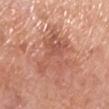Findings:
- notes: catalogued during a skin exam; not biopsied
- TBP lesion metrics: a footprint of about 20 mm², an outline eccentricity of about 0.75 (0 = round, 1 = elongated), and a symmetry-axis asymmetry near 0.5; radial color variation of about 2; a nevus-likeness score of about 0/100
- patient: male, approximately 80 years of age
- location: the left lower leg
- acquisition: 15 mm crop, total-body photography
- tile lighting: white-light illumination
- diameter: ≈7 mm